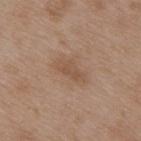{"biopsy_status": "not biopsied; imaged during a skin examination", "lesion_size": {"long_diameter_mm_approx": 3.5}, "patient": {"sex": "male", "age_approx": 50}, "site": "upper back", "lighting": "white-light", "image": {"source": "total-body photography crop", "field_of_view_mm": 15}}